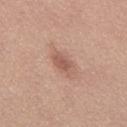About 3.5 mm across.
On the mid back.
Imaged with white-light lighting.
Automated tile analysis of the lesion measured a mean CIELAB color near L≈56 a*≈22 b*≈27 and roughly 10 lightness units darker than nearby skin. It also reported border irregularity of about 2.5 on a 0–10 scale, a color-variation rating of about 2.5/10, and radial color variation of about 1.
The patient is a male aged around 25.
A region of skin cropped from a whole-body photographic capture, roughly 15 mm wide.